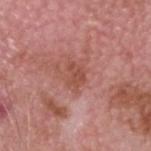Assessment:
Recorded during total-body skin imaging; not selected for excision or biopsy.
Context:
On the head or neck. Captured under white-light illumination. Measured at roughly 3 mm in maximum diameter. A male patient about 75 years old. A 15 mm close-up extracted from a 3D total-body photography capture.